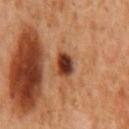Recorded during total-body skin imaging; not selected for excision or biopsy.
The recorded lesion diameter is about 2.5 mm.
This image is a 15 mm lesion crop taken from a total-body photograph.
The lesion is on the mid back.
Automated image analysis of the tile measured a mean CIELAB color near L≈35 a*≈23 b*≈30 and a lesion-to-skin contrast of about 13.5 (normalized; higher = more distinct). The software also gave a border-irregularity rating of about 2/10, a within-lesion color-variation index near 6.5/10, and peripheral color asymmetry of about 2.
A male patient in their 60s.
The tile uses cross-polarized illumination.